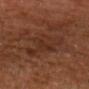No biopsy was performed on this lesion — it was imaged during a full skin examination and was not determined to be concerning. The lesion is on the head or neck. The patient is a male roughly 60 years of age. Imaged with cross-polarized lighting. Automated tile analysis of the lesion measured an area of roughly 14 mm² and a shape eccentricity near 0.65. And it measured an average lesion color of about L≈29 a*≈20 b*≈27 (CIELAB), a lesion–skin lightness drop of about 5, and a normalized border contrast of about 5. Cropped from a total-body skin-imaging series; the visible field is about 15 mm.